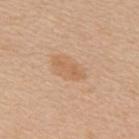The lesion was tiled from a total-body skin photograph and was not biopsied. About 4 mm across. An algorithmic analysis of the crop reported a lesion area of about 4 mm² and a shape eccentricity near 0.95. And it measured roughly 7 lightness units darker than nearby skin and a normalized lesion–skin contrast near 5.5. And it measured border irregularity of about 5 on a 0–10 scale, internal color variation of about 1.5 on a 0–10 scale, and radial color variation of about 0.5. It also reported a classifier nevus-likeness of about 10/100 and lesion-presence confidence of about 100/100. A close-up tile cropped from a whole-body skin photograph, about 15 mm across. A male subject aged approximately 65. The lesion is located on the back. Imaged with white-light lighting.